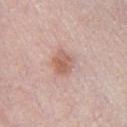Q: Was a biopsy performed?
A: catalogued during a skin exam; not biopsied
Q: What kind of image is this?
A: ~15 mm tile from a whole-body skin photo
Q: What is the lesion's diameter?
A: ~3 mm (longest diameter)
Q: Who is the patient?
A: male, in their 30s